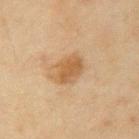<record>
<biopsy_status>not biopsied; imaged during a skin examination</biopsy_status>
</record>